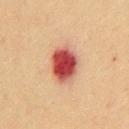Captured during whole-body skin photography for melanoma surveillance; the lesion was not biopsied. Captured under cross-polarized illumination. On the chest. The subject is a male roughly 55 years of age. Automated image analysis of the tile measured a footprint of about 12 mm², a shape eccentricity near 0.65, and a symmetry-axis asymmetry near 0.15. It also reported border irregularity of about 1.5 on a 0–10 scale and a color-variation rating of about 7/10. It also reported a nevus-likeness score of about 0/100 and a detector confidence of about 100 out of 100 that the crop contains a lesion. A region of skin cropped from a whole-body photographic capture, roughly 15 mm wide.